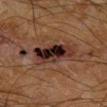Imaged during a routine full-body skin examination; the lesion was not biopsied and no histopathology is available. A region of skin cropped from a whole-body photographic capture, roughly 15 mm wide. The patient is a male aged around 65. The lesion is on the leg. Automated tile analysis of the lesion measured a mean CIELAB color near L≈20 a*≈16 b*≈17 and about 14 CIELAB-L* units darker than the surrounding skin. The software also gave a border-irregularity rating of about 3/10 and internal color variation of about 10 on a 0–10 scale.